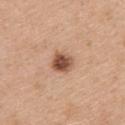follow-up: total-body-photography surveillance lesion; no biopsy
TBP lesion metrics: about 16 CIELAB-L* units darker than the surrounding skin; a border-irregularity rating of about 1.5/10, a color-variation rating of about 5/10, and a peripheral color-asymmetry measure near 1.5; an automated nevus-likeness rating near 95 out of 100
lighting: white-light
acquisition: total-body-photography crop, ~15 mm field of view
patient: female, in their mid- to late 40s
site: the right upper arm